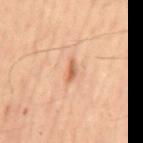Clinical impression:
Imaged during a routine full-body skin examination; the lesion was not biopsied and no histopathology is available.
Image and clinical context:
Measured at roughly 2.5 mm in maximum diameter. A roughly 15 mm field-of-view crop from a total-body skin photograph. A male patient about 65 years old. The lesion is located on the back. An algorithmic analysis of the crop reported an area of roughly 2.5 mm², an outline eccentricity of about 0.9 (0 = round, 1 = elongated), and a symmetry-axis asymmetry near 0.35. The software also gave a mean CIELAB color near L≈61 a*≈24 b*≈36, roughly 11 lightness units darker than nearby skin, and a lesion-to-skin contrast of about 7.5 (normalized; higher = more distinct). The analysis additionally found a border-irregularity rating of about 3.5/10, internal color variation of about 0 on a 0–10 scale, and peripheral color asymmetry of about 0. Imaged with cross-polarized lighting.